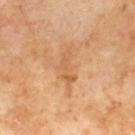notes = imaged on a skin check; not biopsied
size = ~4.5 mm (longest diameter)
anatomic site = the upper back
automated metrics = an average lesion color of about L≈59 a*≈22 b*≈39 (CIELAB), roughly 7 lightness units darker than nearby skin, and a normalized lesion–skin contrast near 5; a color-variation rating of about 2/10 and radial color variation of about 0
illumination = cross-polarized illumination
image source = total-body-photography crop, ~15 mm field of view
patient = male, aged 68–72